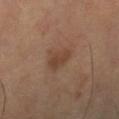Captured during whole-body skin photography for melanoma surveillance; the lesion was not biopsied.
Cropped from a whole-body photographic skin survey; the tile spans about 15 mm.
The lesion is on the leg.
The tile uses cross-polarized illumination.
The subject is a female aged 58–62.
The total-body-photography lesion software estimated a shape-asymmetry score of about 0.2 (0 = symmetric). The software also gave a color-variation rating of about 2/10 and radial color variation of about 0.5.